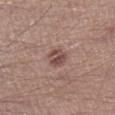follow-up: total-body-photography surveillance lesion; no biopsy | subject: male, aged 38–42 | image: ~15 mm crop, total-body skin-cancer survey | automated lesion analysis: an area of roughly 5 mm², an outline eccentricity of about 0.5 (0 = round, 1 = elongated), and two-axis asymmetry of about 0.15; a mean CIELAB color near L≈48 a*≈19 b*≈22, a lesion–skin lightness drop of about 11, and a normalized border contrast of about 8; a border-irregularity rating of about 1.5/10 and a peripheral color-asymmetry measure near 2; an automated nevus-likeness rating near 30 out of 100 | location: the right lower leg | tile lighting: white-light | size: about 2.5 mm.